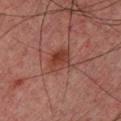Captured during whole-body skin photography for melanoma surveillance; the lesion was not biopsied.
Automated image analysis of the tile measured a lesion color around L≈32 a*≈22 b*≈23 in CIELAB, roughly 7 lightness units darker than nearby skin, and a normalized lesion–skin contrast near 7.5. The analysis additionally found a detector confidence of about 100 out of 100 that the crop contains a lesion.
A male patient, aged approximately 30.
A region of skin cropped from a whole-body photographic capture, roughly 15 mm wide.
The lesion is located on the chest.
The recorded lesion diameter is about 3 mm.